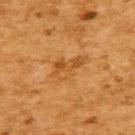Assessment: The lesion was photographed on a routine skin check and not biopsied; there is no pathology result.